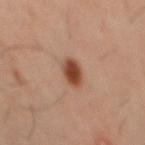workup = imaged on a skin check; not biopsied
lesion size = about 3.5 mm
acquisition = ~15 mm tile from a whole-body skin photo
automated metrics = an area of roughly 6 mm², a shape eccentricity near 0.8, and a symmetry-axis asymmetry near 0.1; about 14 CIELAB-L* units darker than the surrounding skin and a normalized border contrast of about 11
illumination = cross-polarized illumination
anatomic site = the mid back
patient = male, in their 40s